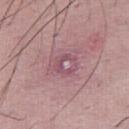Imaged during a routine full-body skin examination; the lesion was not biopsied and no histopathology is available.
A male subject roughly 55 years of age.
The lesion is located on the right thigh.
A 15 mm close-up extracted from a 3D total-body photography capture.
The lesion's longest dimension is about 3.5 mm.
This is a white-light tile.